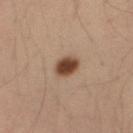The lesion was photographed on a routine skin check and not biopsied; there is no pathology result. The total-body-photography lesion software estimated a footprint of about 5.5 mm², an outline eccentricity of about 0.6 (0 = round, 1 = elongated), and a symmetry-axis asymmetry near 0.2. It also reported border irregularity of about 1.5 on a 0–10 scale, a within-lesion color-variation index near 3/10, and a peripheral color-asymmetry measure near 1. And it measured a classifier nevus-likeness of about 100/100 and a detector confidence of about 100 out of 100 that the crop contains a lesion. A male subject aged around 35. The recorded lesion diameter is about 3 mm. This is a cross-polarized tile. From the right upper arm. A 15 mm close-up tile from a total-body photography series done for melanoma screening.